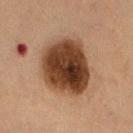The lesion was photographed on a routine skin check and not biopsied; there is no pathology result. Located on the back. The lesion-visualizer software estimated an average lesion color of about L≈28 a*≈16 b*≈24 (CIELAB), roughly 16 lightness units darker than nearby skin, and a lesion-to-skin contrast of about 14.5 (normalized; higher = more distinct). The software also gave a classifier nevus-likeness of about 95/100 and lesion-presence confidence of about 100/100. A 15 mm close-up extracted from a 3D total-body photography capture. The recorded lesion diameter is about 6.5 mm. A female subject, aged around 80.